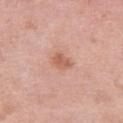biopsy_status: not biopsied; imaged during a skin examination
site: left thigh
image:
  source: total-body photography crop
  field_of_view_mm: 15
patient:
  sex: female
  age_approx: 40